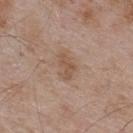Imaged during a routine full-body skin examination; the lesion was not biopsied and no histopathology is available. A male patient, about 55 years old. A lesion tile, about 15 mm wide, cut from a 3D total-body photograph. The lesion is located on the upper back. Longest diameter approximately 3 mm.